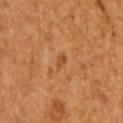Imaged during a routine full-body skin examination; the lesion was not biopsied and no histopathology is available. An algorithmic analysis of the crop reported a border-irregularity index near 2/10, internal color variation of about 2.5 on a 0–10 scale, and radial color variation of about 1. It also reported a classifier nevus-likeness of about 0/100 and a detector confidence of about 100 out of 100 that the crop contains a lesion. A 15 mm close-up tile from a total-body photography series done for melanoma screening. The lesion's longest dimension is about 2.5 mm. Captured under cross-polarized illumination. A female patient, aged 48 to 52. The lesion is located on the arm.